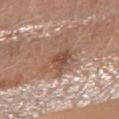{
  "biopsy_status": "not biopsied; imaged during a skin examination",
  "automated_metrics": {
    "vs_skin_contrast_norm": 6.5,
    "border_irregularity_0_10": 5.0
  },
  "patient": {
    "sex": "female",
    "age_approx": 55
  },
  "lighting": "white-light",
  "image": {
    "source": "total-body photography crop",
    "field_of_view_mm": 15
  },
  "site": "right forearm",
  "lesion_size": {
    "long_diameter_mm_approx": 5.0
  }
}